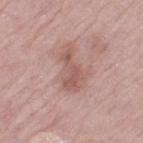Part of a total-body skin-imaging series; this lesion was reviewed on a skin check and was not flagged for biopsy.
Longest diameter approximately 5 mm.
The lesion is on the left thigh.
A roughly 15 mm field-of-view crop from a total-body skin photograph.
Captured under white-light illumination.
A female patient, in their 70s.